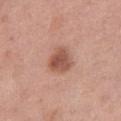image source=~15 mm tile from a whole-body skin photo; patient=female, aged 48 to 52; body site=the right thigh.